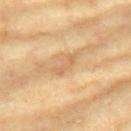{
  "biopsy_status": "not biopsied; imaged during a skin examination",
  "patient": {
    "sex": "female",
    "age_approx": 75
  },
  "automated_metrics": {
    "area_mm2_approx": 3.5,
    "eccentricity": 0.9,
    "shape_asymmetry": 0.45,
    "cielab_L": 66,
    "cielab_a": 19,
    "cielab_b": 40,
    "vs_skin_darker_L": 8.0,
    "vs_skin_contrast_norm": 5.0,
    "nevus_likeness_0_100": 0,
    "lesion_detection_confidence_0_100": 85
  },
  "lighting": "cross-polarized",
  "image": {
    "source": "total-body photography crop",
    "field_of_view_mm": 15
  },
  "lesion_size": {
    "long_diameter_mm_approx": 3.0
  },
  "site": "right upper arm"
}